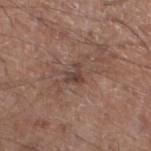notes — imaged on a skin check; not biopsied
location — the leg
automated metrics — a footprint of about 3 mm², an outline eccentricity of about 0.65 (0 = round, 1 = elongated), and two-axis asymmetry of about 0.6; about 8 CIELAB-L* units darker than the surrounding skin; a border-irregularity rating of about 6.5/10, internal color variation of about 1.5 on a 0–10 scale, and a peripheral color-asymmetry measure near 0.5; a detector confidence of about 100 out of 100 that the crop contains a lesion
patient — male, about 60 years old
diameter — ~2.5 mm (longest diameter)
illumination — white-light illumination
image — total-body-photography crop, ~15 mm field of view